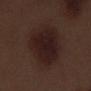Notes:
• biopsy status · catalogued during a skin exam; not biopsied
• lesion size · ≈7.5 mm
• subject · male, in their 70s
• site · the right thigh
• image source · ~15 mm tile from a whole-body skin photo
• illumination · white-light illumination